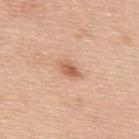No biopsy was performed on this lesion — it was imaged during a full skin examination and was not determined to be concerning. A male subject, approximately 50 years of age. The tile uses white-light illumination. Approximately 3 mm at its widest. From the upper back. A lesion tile, about 15 mm wide, cut from a 3D total-body photograph.